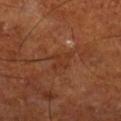Acquisition and patient details: The subject is a male aged approximately 65. The lesion-visualizer software estimated an average lesion color of about L≈33 a*≈23 b*≈30 (CIELAB) and about 5 CIELAB-L* units darker than the surrounding skin. And it measured a within-lesion color-variation index near 1/10 and radial color variation of about 0.5. This is a cross-polarized tile. Cropped from a total-body skin-imaging series; the visible field is about 15 mm. On the right lower leg. The lesion's longest dimension is about 3 mm.